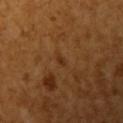Recorded during total-body skin imaging; not selected for excision or biopsy.